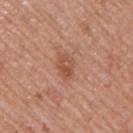Case summary:
• notes · catalogued during a skin exam; not biopsied
• subject · male, in their mid- to late 50s
• lesion diameter · ≈3 mm
• anatomic site · the right upper arm
• image source · ~15 mm tile from a whole-body skin photo